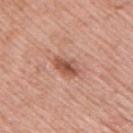Case summary:
• anatomic site · the upper back
• acquisition · ~15 mm tile from a whole-body skin photo
• subject · male, in their mid- to late 50s
• automated metrics · an area of roughly 5 mm², an outline eccentricity of about 0.85 (0 = round, 1 = elongated), and a symmetry-axis asymmetry near 0.25; a classifier nevus-likeness of about 75/100 and a lesion-detection confidence of about 100/100